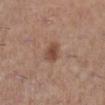The lesion is on the right lower leg.
A female patient, about 45 years old.
Cropped from a total-body skin-imaging series; the visible field is about 15 mm.